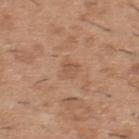Impression: Imaged during a routine full-body skin examination; the lesion was not biopsied and no histopathology is available. Acquisition and patient details: A male subject in their 40s. Longest diameter approximately 2.5 mm. The tile uses white-light illumination. The lesion is on the upper back. A 15 mm crop from a total-body photograph taken for skin-cancer surveillance.